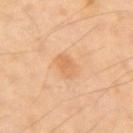notes: total-body-photography surveillance lesion; no biopsy
body site: the right upper arm
patient: male, about 70 years old
image: ~15 mm crop, total-body skin-cancer survey